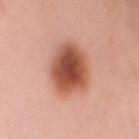Measured at roughly 5.5 mm in maximum diameter. The patient is a female roughly 50 years of age. This is a white-light tile. A roughly 15 mm field-of-view crop from a total-body skin photograph. From the front of the torso.